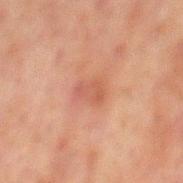Case summary:
• biopsy status: imaged on a skin check; not biopsied
• body site: the mid back
• subject: male, aged around 60
• lesion size: about 2.5 mm
• lighting: cross-polarized
• TBP lesion metrics: an area of roughly 4.5 mm² and an eccentricity of roughly 0.65; an average lesion color of about L≈42 a*≈21 b*≈26 (CIELAB), about 6 CIELAB-L* units darker than the surrounding skin, and a normalized border contrast of about 5; a border-irregularity rating of about 3.5/10, a within-lesion color-variation index near 2/10, and a peripheral color-asymmetry measure near 0.5
• acquisition: ~15 mm tile from a whole-body skin photo